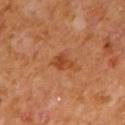Recorded during total-body skin imaging; not selected for excision or biopsy. Captured under cross-polarized illumination. A region of skin cropped from a whole-body photographic capture, roughly 15 mm wide. The lesion-visualizer software estimated a lesion area of about 3.5 mm², an eccentricity of roughly 0.7, and a shape-asymmetry score of about 0.35 (0 = symmetric). The analysis additionally found a lesion color around L≈43 a*≈27 b*≈38 in CIELAB, about 8 CIELAB-L* units darker than the surrounding skin, and a normalized lesion–skin contrast near 7. On the mid back. The recorded lesion diameter is about 2.5 mm. A male subject, in their mid- to late 60s.